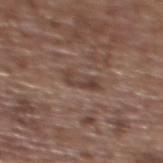Impression: The lesion was photographed on a routine skin check and not biopsied; there is no pathology result. Background: On the upper back. A 15 mm close-up tile from a total-body photography series done for melanoma screening. The subject is a female aged 63–67. The tile uses white-light illumination.